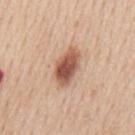Q: Was this lesion biopsied?
A: catalogued during a skin exam; not biopsied
Q: Automated lesion metrics?
A: a border-irregularity rating of about 2/10, a within-lesion color-variation index near 5/10, and radial color variation of about 1.5; a lesion-detection confidence of about 100/100
Q: What is the lesion's diameter?
A: ~4.5 mm (longest diameter)
Q: Where on the body is the lesion?
A: the mid back
Q: What are the patient's age and sex?
A: male, roughly 60 years of age
Q: What kind of image is this?
A: ~15 mm crop, total-body skin-cancer survey